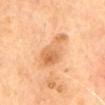Q: What lighting was used for the tile?
A: cross-polarized
Q: How was this image acquired?
A: total-body-photography crop, ~15 mm field of view
Q: What is the lesion's diameter?
A: ~6 mm (longest diameter)
Q: What is the anatomic site?
A: the mid back
Q: Patient demographics?
A: male, aged 68–72